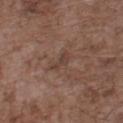Assessment:
This lesion was catalogued during total-body skin photography and was not selected for biopsy.
Context:
Approximately 2.5 mm at its widest. Located on the front of the torso. Automated tile analysis of the lesion measured an area of roughly 3 mm², a shape eccentricity near 0.9, and a symmetry-axis asymmetry near 0.4. The software also gave a mean CIELAB color near L≈40 a*≈17 b*≈23, a lesion–skin lightness drop of about 6, and a normalized border contrast of about 5.5. It also reported a nevus-likeness score of about 0/100 and a lesion-detection confidence of about 95/100. A lesion tile, about 15 mm wide, cut from a 3D total-body photograph. A male patient, aged 68–72. Imaged with white-light lighting.